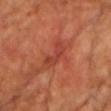Assessment:
The lesion was tiled from a total-body skin photograph and was not biopsied.
Clinical summary:
Approximately 4.5 mm at its widest. A male subject, aged 58–62. Imaged with cross-polarized lighting. On the chest. A lesion tile, about 15 mm wide, cut from a 3D total-body photograph.